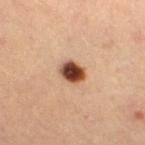workup: no biopsy performed (imaged during a skin exam); site: the leg; diameter: about 3 mm; imaging modality: total-body-photography crop, ~15 mm field of view; patient: female, aged around 45; illumination: cross-polarized illumination.